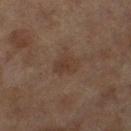notes=total-body-photography surveillance lesion; no biopsy | body site=the left thigh | illumination=cross-polarized | patient=female, aged 58–62 | imaging modality=~15 mm tile from a whole-body skin photo.